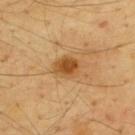Impression: Recorded during total-body skin imaging; not selected for excision or biopsy. Background: An algorithmic analysis of the crop reported a mean CIELAB color near L≈52 a*≈22 b*≈43, about 12 CIELAB-L* units darker than the surrounding skin, and a normalized lesion–skin contrast near 8.5. And it measured a nevus-likeness score of about 95/100 and a detector confidence of about 100 out of 100 that the crop contains a lesion. The lesion's longest dimension is about 3.5 mm. This image is a 15 mm lesion crop taken from a total-body photograph. The lesion is on the upper back. A male subject in their mid-60s. Imaged with cross-polarized lighting.